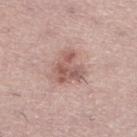Imaged during a routine full-body skin examination; the lesion was not biopsied and no histopathology is available. Approximately 3.5 mm at its widest. A close-up tile cropped from a whole-body skin photograph, about 15 mm across. The lesion is on the left lower leg. An algorithmic analysis of the crop reported an outline eccentricity of about 0.4 (0 = round, 1 = elongated) and two-axis asymmetry of about 0.4. It also reported a lesion color around L≈57 a*≈21 b*≈24 in CIELAB, roughly 11 lightness units darker than nearby skin, and a lesion-to-skin contrast of about 7 (normalized; higher = more distinct). A female subject, aged 38 to 42.